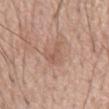notes: catalogued during a skin exam; not biopsied | body site: the mid back | tile lighting: white-light illumination | imaging modality: ~15 mm tile from a whole-body skin photo | subject: male, in their mid-60s | diameter: about 3 mm.